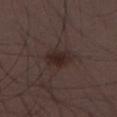Cropped from a total-body skin-imaging series; the visible field is about 15 mm.
Automated tile analysis of the lesion measured a lesion color around L≈25 a*≈14 b*≈17 in CIELAB, roughly 7 lightness units darker than nearby skin, and a lesion-to-skin contrast of about 8.5 (normalized; higher = more distinct). It also reported a border-irregularity rating of about 2.5/10, internal color variation of about 3.5 on a 0–10 scale, and radial color variation of about 1.5.
Located on the left thigh.
A male patient, aged approximately 30.
Imaged with white-light lighting.
About 3.5 mm across.